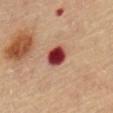Recorded during total-body skin imaging; not selected for excision or biopsy. Captured under cross-polarized illumination. A roughly 15 mm field-of-view crop from a total-body skin photograph. The patient is a male roughly 85 years of age or older. The lesion is located on the chest.